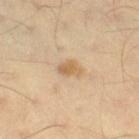notes: imaged on a skin check; not biopsied | tile lighting: cross-polarized illumination | anatomic site: the right thigh | imaging modality: ~15 mm crop, total-body skin-cancer survey | patient: male, about 40 years old | TBP lesion metrics: a footprint of about 4 mm², an outline eccentricity of about 0.75 (0 = round, 1 = elongated), and two-axis asymmetry of about 0.35; an average lesion color of about L≈63 a*≈17 b*≈37 (CIELAB), roughly 9 lightness units darker than nearby skin, and a lesion-to-skin contrast of about 7 (normalized; higher = more distinct); border irregularity of about 3 on a 0–10 scale and a within-lesion color-variation index near 1.5/10; a classifier nevus-likeness of about 40/100 and a detector confidence of about 100 out of 100 that the crop contains a lesion | diameter: about 2.5 mm.